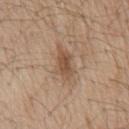Case summary:
* biopsy status — catalogued during a skin exam; not biopsied
* lighting — white-light illumination
* image — ~15 mm crop, total-body skin-cancer survey
* patient — male, about 70 years old
* TBP lesion metrics — an area of roughly 7 mm² and a shape eccentricity near 0.8; a mean CIELAB color near L≈52 a*≈17 b*≈30 and roughly 10 lightness units darker than nearby skin; a border-irregularity index near 3.5/10, a within-lesion color-variation index near 3/10, and radial color variation of about 1
* lesion size — ~4 mm (longest diameter)
* site — the chest